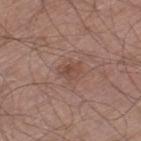Notes:
* notes · total-body-photography surveillance lesion; no biopsy